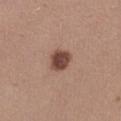Notes:
• notes — catalogued during a skin exam; not biopsied
• acquisition — ~15 mm tile from a whole-body skin photo
• location — the right thigh
• subject — female, in their mid- to late 40s